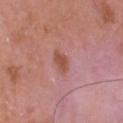Clinical summary:
A male subject, about 50 years old. Captured under white-light illumination. The lesion's longest dimension is about 2.5 mm. The lesion is on the head or neck. A lesion tile, about 15 mm wide, cut from a 3D total-body photograph.